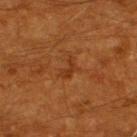The lesion was tiled from a total-body skin photograph and was not biopsied.
A male subject, aged approximately 60.
Captured under cross-polarized illumination.
Cropped from a total-body skin-imaging series; the visible field is about 15 mm.
On the back.
Automated tile analysis of the lesion measured a mean CIELAB color near L≈33 a*≈24 b*≈35, roughly 6 lightness units darker than nearby skin, and a normalized lesion–skin contrast near 6. And it measured an automated nevus-likeness rating near 0 out of 100 and lesion-presence confidence of about 100/100.
The recorded lesion diameter is about 2.5 mm.